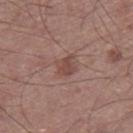notes = imaged on a skin check; not biopsied | patient = male, aged around 60 | site = the right thigh | illumination = white-light illumination | image = 15 mm crop, total-body photography | size = about 3 mm.